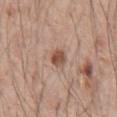<tbp_lesion>
<biopsy_status>not biopsied; imaged during a skin examination</biopsy_status>
<automated_metrics>
  <area_mm2_approx>4.0</area_mm2_approx>
  <eccentricity>0.7</eccentricity>
  <shape_asymmetry>0.25</shape_asymmetry>
  <cielab_L>51</cielab_L>
  <cielab_a>20</cielab_a>
  <cielab_b>28</cielab_b>
  <vs_skin_darker_L>12.0</vs_skin_darker_L>
  <vs_skin_contrast_norm>8.5</vs_skin_contrast_norm>
  <border_irregularity_0_10>2.5</border_irregularity_0_10>
  <color_variation_0_10>3.5</color_variation_0_10>
  <peripheral_color_asymmetry>1.0</peripheral_color_asymmetry>
</automated_metrics>
<lighting>white-light</lighting>
<image>
  <source>total-body photography crop</source>
  <field_of_view_mm>15</field_of_view_mm>
</image>
<patient>
  <sex>male</sex>
  <age_approx>55</age_approx>
</patient>
<site>chest</site>
</tbp_lesion>